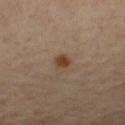Context: The patient is a male aged 63 to 67. This is a cross-polarized tile. A roughly 15 mm field-of-view crop from a total-body skin photograph. Located on the left forearm. The lesion's longest dimension is about 2 mm.